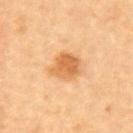<record>
<biopsy_status>not biopsied; imaged during a skin examination</biopsy_status>
<site>left upper arm</site>
<patient>
  <sex>male</sex>
  <age_approx>85+</age_approx>
</patient>
<lesion_size>
  <long_diameter_mm_approx>3.5</long_diameter_mm_approx>
</lesion_size>
<image>
  <source>total-body photography crop</source>
  <field_of_view_mm>15</field_of_view_mm>
</image>
<automated_metrics>
  <border_irregularity_0_10>2.0</border_irregularity_0_10>
  <peripheral_color_asymmetry>1.5</peripheral_color_asymmetry>
</automated_metrics>
</record>